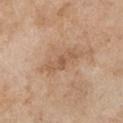This lesion was catalogued during total-body skin photography and was not selected for biopsy.